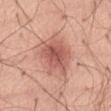Case summary:
• workup — total-body-photography surveillance lesion; no biopsy
• image source — 15 mm crop, total-body photography
• patient — male, aged 68 to 72
• body site — the abdomen
• diameter — ≈5.5 mm
• illumination — white-light